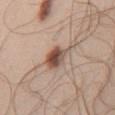Part of a total-body skin-imaging series; this lesion was reviewed on a skin check and was not flagged for biopsy.
Automated image analysis of the tile measured a classifier nevus-likeness of about 100/100 and a lesion-detection confidence of about 100/100.
Cropped from a total-body skin-imaging series; the visible field is about 15 mm.
Located on the chest.
About 4 mm across.
A male subject, aged 53 to 57.